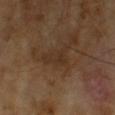This lesion was catalogued during total-body skin photography and was not selected for biopsy.
The recorded lesion diameter is about 4 mm.
Automated tile analysis of the lesion measured a lesion area of about 6.5 mm², an outline eccentricity of about 0.8 (0 = round, 1 = elongated), and a shape-asymmetry score of about 0.45 (0 = symmetric). The analysis additionally found a mean CIELAB color near L≈30 a*≈15 b*≈26, roughly 5 lightness units darker than nearby skin, and a normalized lesion–skin contrast near 5.5. The analysis additionally found a within-lesion color-variation index near 2/10 and a peripheral color-asymmetry measure near 0.5. The software also gave an automated nevus-likeness rating near 0 out of 100.
A male patient, in their mid- to late 60s.
A 15 mm close-up tile from a total-body photography series done for melanoma screening.
This is a cross-polarized tile.